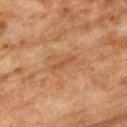Clinical impression:
Part of a total-body skin-imaging series; this lesion was reviewed on a skin check and was not flagged for biopsy.
Acquisition and patient details:
A male patient about 80 years old. From the left upper arm. Captured under cross-polarized illumination. Automated image analysis of the tile measured a footprint of about 2 mm² and two-axis asymmetry of about 0.35. And it measured a mean CIELAB color near L≈39 a*≈19 b*≈30, roughly 6 lightness units darker than nearby skin, and a normalized lesion–skin contrast near 5.5. A roughly 15 mm field-of-view crop from a total-body skin photograph.